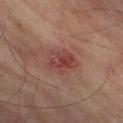Q: Was a biopsy performed?
A: imaged on a skin check; not biopsied
Q: What is the lesion's diameter?
A: about 4 mm
Q: Automated lesion metrics?
A: an average lesion color of about L≈35 a*≈21 b*≈20 (CIELAB), a lesion–skin lightness drop of about 7, and a lesion-to-skin contrast of about 6.5 (normalized; higher = more distinct); a border-irregularity rating of about 2.5/10; a nevus-likeness score of about 5/100 and a detector confidence of about 100 out of 100 that the crop contains a lesion
Q: Where on the body is the lesion?
A: the right thigh
Q: What lighting was used for the tile?
A: cross-polarized illumination
Q: How was this image acquired?
A: total-body-photography crop, ~15 mm field of view
Q: What are the patient's age and sex?
A: male, aged approximately 75